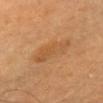The lesion was tiled from a total-body skin photograph and was not biopsied. About 4.5 mm across. This image is a 15 mm lesion crop taken from a total-body photograph. A female subject, aged around 60. Located on the head or neck.